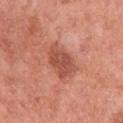Impression: This lesion was catalogued during total-body skin photography and was not selected for biopsy.